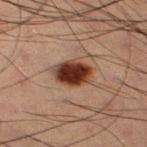• follow-up · total-body-photography surveillance lesion; no biopsy
• anatomic site · the right thigh
• patient · male, aged around 55
• image source · ~15 mm tile from a whole-body skin photo